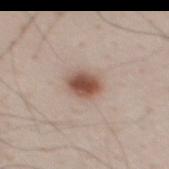Q: Who is the patient?
A: male, approximately 35 years of age
Q: What did automated image analysis measure?
A: a lesion area of about 7 mm², an eccentricity of roughly 0.65, and a shape-asymmetry score of about 0.15 (0 = symmetric)
Q: How large is the lesion?
A: ≈3.5 mm
Q: How was this image acquired?
A: ~15 mm crop, total-body skin-cancer survey
Q: What is the anatomic site?
A: the mid back